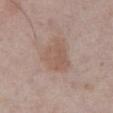size: about 4.5 mm | anatomic site: the abdomen | image: total-body-photography crop, ~15 mm field of view | patient: male, aged around 75.